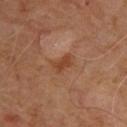Captured during whole-body skin photography for melanoma surveillance; the lesion was not biopsied.
Approximately 2.5 mm at its widest.
The lesion is located on the upper back.
A 15 mm close-up extracted from a 3D total-body photography capture.
A male patient, in their mid-60s.